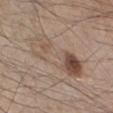Findings:
* biopsy status: no biopsy performed (imaged during a skin exam)
* illumination: white-light
* patient: male, roughly 45 years of age
* automated lesion analysis: a lesion color around L≈52 a*≈15 b*≈25 in CIELAB, roughly 8 lightness units darker than nearby skin, and a normalized border contrast of about 5.5; a border-irregularity rating of about 3.5/10, a color-variation rating of about 10/10, and peripheral color asymmetry of about 4.5
* lesion size: ≈8 mm
* body site: the leg
* imaging modality: 15 mm crop, total-body photography